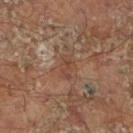Clinical summary:
Located on the right lower leg. Measured at roughly 3 mm in maximum diameter. A male patient, in their mid- to late 60s. A close-up tile cropped from a whole-body skin photograph, about 15 mm across. Automated tile analysis of the lesion measured roughly 6 lightness units darker than nearby skin and a lesion-to-skin contrast of about 5.5 (normalized; higher = more distinct). And it measured a border-irregularity index near 3/10, a color-variation rating of about 2/10, and peripheral color asymmetry of about 0.5. Captured under cross-polarized illumination.